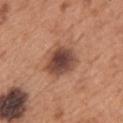Q: Was this lesion biopsied?
A: no biopsy performed (imaged during a skin exam)
Q: Lesion location?
A: the upper back
Q: How was this image acquired?
A: total-body-photography crop, ~15 mm field of view
Q: Patient demographics?
A: male, aged approximately 65